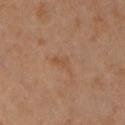{"biopsy_status": "not biopsied; imaged during a skin examination", "lesion_size": {"long_diameter_mm_approx": 2.5}, "lighting": "cross-polarized", "image": {"source": "total-body photography crop", "field_of_view_mm": 15}, "patient": {"sex": "male", "age_approx": 40}, "automated_metrics": {"eccentricity": 0.8, "shape_asymmetry": 0.45, "cielab_L": 49, "cielab_a": 19, "cielab_b": 32, "vs_skin_darker_L": 5.0, "vs_skin_contrast_norm": 4.5, "nevus_likeness_0_100": 0}, "site": "front of the torso"}